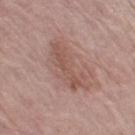notes = total-body-photography surveillance lesion; no biopsy | diameter = ≈6.5 mm | patient = female, aged approximately 85 or older | imaging modality = ~15 mm tile from a whole-body skin photo | location = the left thigh | lighting = white-light illumination.